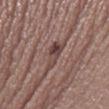Imaged during a routine full-body skin examination; the lesion was not biopsied and no histopathology is available.
The patient is a female aged around 30.
From the right thigh.
A lesion tile, about 15 mm wide, cut from a 3D total-body photograph.
The tile uses white-light illumination.